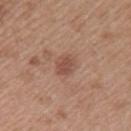biopsy status=catalogued during a skin exam; not biopsied | image=total-body-photography crop, ~15 mm field of view | site=the left upper arm | lesion diameter=~2.5 mm (longest diameter) | tile lighting=white-light | subject=female, about 40 years old.